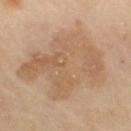Part of a total-body skin-imaging series; this lesion was reviewed on a skin check and was not flagged for biopsy.
Captured under cross-polarized illumination.
The lesion's longest dimension is about 10 mm.
A close-up tile cropped from a whole-body skin photograph, about 15 mm across.
The lesion is located on the right thigh.
A male patient aged 63–67.